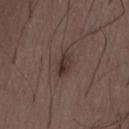Assessment: This lesion was catalogued during total-body skin photography and was not selected for biopsy. Clinical summary: The patient is a male in their 50s. A close-up tile cropped from a whole-body skin photograph, about 15 mm across. The tile uses white-light illumination. Located on the abdomen. Measured at roughly 3.5 mm in maximum diameter. The total-body-photography lesion software estimated an average lesion color of about L≈33 a*≈15 b*≈17 (CIELAB) and a normalized lesion–skin contrast near 8. And it measured a classifier nevus-likeness of about 55/100 and a lesion-detection confidence of about 100/100.